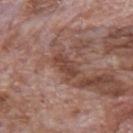Notes:
• follow-up · imaged on a skin check; not biopsied
• body site · the back
• image · ~15 mm tile from a whole-body skin photo
• patient · male, aged 68 to 72
• lesion size · about 3.5 mm
• automated lesion analysis · internal color variation of about 2.5 on a 0–10 scale and a peripheral color-asymmetry measure near 1; lesion-presence confidence of about 95/100